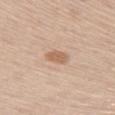<case>
<biopsy_status>not biopsied; imaged during a skin examination</biopsy_status>
<lesion_size>
  <long_diameter_mm_approx>3.0</long_diameter_mm_approx>
</lesion_size>
<lighting>white-light</lighting>
<site>left thigh</site>
<patient>
  <sex>male</sex>
  <age_approx>60</age_approx>
</patient>
<image>
  <source>total-body photography crop</source>
  <field_of_view_mm>15</field_of_view_mm>
</image>
</case>